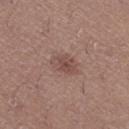| field | value |
|---|---|
| tile lighting | white-light |
| location | the right thigh |
| acquisition | total-body-photography crop, ~15 mm field of view |
| subject | female, aged 23–27 |
| lesion diameter | about 3 mm |